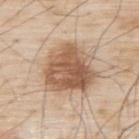Findings:
– biopsy status — no biopsy performed (imaged during a skin exam)
– acquisition — total-body-photography crop, ~15 mm field of view
– lighting — white-light
– image-analysis metrics — roughly 14 lightness units darker than nearby skin and a normalized lesion–skin contrast near 9; internal color variation of about 6.5 on a 0–10 scale and peripheral color asymmetry of about 2
– body site — the upper back
– patient — male, about 80 years old
– diameter — ≈6.5 mm